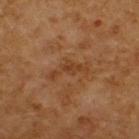  image:
    source: total-body photography crop
    field_of_view_mm: 15
  site: upper back
  lesion_size:
    long_diameter_mm_approx: 5.0
  patient:
    sex: male
    age_approx: 60